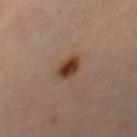- follow-up: imaged on a skin check; not biopsied
- automated lesion analysis: a mean CIELAB color near L≈38 a*≈20 b*≈28, a lesion–skin lightness drop of about 13, and a normalized border contrast of about 11; a border-irregularity rating of about 1.5/10 and a color-variation rating of about 4/10; an automated nevus-likeness rating near 100 out of 100 and a lesion-detection confidence of about 100/100
- site: the right thigh
- subject: female, aged 33 to 37
- image source: 15 mm crop, total-body photography
- tile lighting: cross-polarized
- size: ~3 mm (longest diameter)